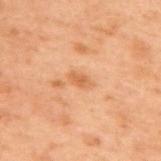<tbp_lesion>
  <biopsy_status>not biopsied; imaged during a skin examination</biopsy_status>
  <image>
    <source>total-body photography crop</source>
    <field_of_view_mm>15</field_of_view_mm>
  </image>
  <lighting>cross-polarized</lighting>
  <patient>
    <sex>male</sex>
    <age_approx>70</age_approx>
  </patient>
  <site>upper back</site>
  <automated_metrics>
    <area_mm2_approx>3.5</area_mm2_approx>
    <eccentricity>0.85</eccentricity>
    <shape_asymmetry>0.2</shape_asymmetry>
    <border_irregularity_0_10>2.0</border_irregularity_0_10>
    <color_variation_0_10>2.0</color_variation_0_10>
    <peripheral_color_asymmetry>0.5</peripheral_color_asymmetry>
  </automated_metrics>
</tbp_lesion>